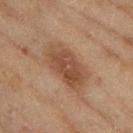Clinical impression: Part of a total-body skin-imaging series; this lesion was reviewed on a skin check and was not flagged for biopsy. Background: The patient is a female in their 60s. A roughly 15 mm field-of-view crop from a total-body skin photograph. The lesion is on the left thigh.